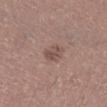Imaged during a routine full-body skin examination; the lesion was not biopsied and no histopathology is available. An algorithmic analysis of the crop reported a footprint of about 4.5 mm² and a shape eccentricity near 0.7. And it measured an average lesion color of about L≈50 a*≈18 b*≈22 (CIELAB), about 8 CIELAB-L* units darker than the surrounding skin, and a normalized border contrast of about 6. The software also gave a nevus-likeness score of about 5/100 and lesion-presence confidence of about 100/100. On the leg. Cropped from a whole-body photographic skin survey; the tile spans about 15 mm. The patient is a male aged approximately 45.